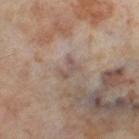No biopsy was performed on this lesion — it was imaged during a full skin examination and was not determined to be concerning. A roughly 15 mm field-of-view crop from a total-body skin photograph. The lesion-visualizer software estimated an area of roughly 3.5 mm² and a shape-asymmetry score of about 0.4 (0 = symmetric). The software also gave border irregularity of about 4.5 on a 0–10 scale and peripheral color asymmetry of about 0. The software also gave lesion-presence confidence of about 85/100. Captured under cross-polarized illumination. The lesion is on the right thigh. A female patient approximately 55 years of age.